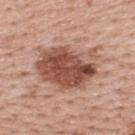biopsy_status: not biopsied; imaged during a skin examination
image:
  source: total-body photography crop
  field_of_view_mm: 15
lighting: white-light
lesion_size:
  long_diameter_mm_approx: 7.0
automated_metrics:
  vs_skin_darker_L: 15.0
  vs_skin_contrast_norm: 10.0
  border_irregularity_0_10: 3.0
  color_variation_0_10: 7.5
  peripheral_color_asymmetry: 3.0
  nevus_likeness_0_100: 95
  lesion_detection_confidence_0_100: 100
site: back
patient:
  sex: male
  age_approx: 40